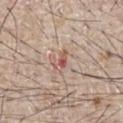Automated tile analysis of the lesion measured an area of roughly 3 mm², an outline eccentricity of about 0.85 (0 = round, 1 = elongated), and two-axis asymmetry of about 0.45. It also reported a nevus-likeness score of about 0/100 and a lesion-detection confidence of about 100/100. Longest diameter approximately 2.5 mm. On the chest. The tile uses white-light illumination. A 15 mm close-up tile from a total-body photography series done for melanoma screening. A male subject, aged approximately 65.